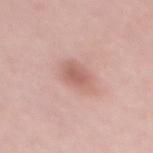  image:
    source: total-body photography crop
    field_of_view_mm: 15
  patient:
    sex: female
    age_approx: 60
  site: mid back